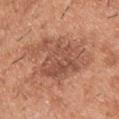This image is a 15 mm lesion crop taken from a total-body photograph. Captured under white-light illumination. A male patient about 40 years old. The lesion's longest dimension is about 8.5 mm. The lesion is on the front of the torso.